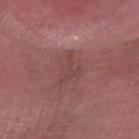Assessment: Recorded during total-body skin imaging; not selected for excision or biopsy. Image and clinical context: The lesion is located on the left forearm. A 15 mm close-up extracted from a 3D total-body photography capture. A male patient, aged 63 to 67.